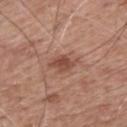Assessment: Recorded during total-body skin imaging; not selected for excision or biopsy. Background: A lesion tile, about 15 mm wide, cut from a 3D total-body photograph. A male subject, approximately 60 years of age. Captured under white-light illumination. The total-body-photography lesion software estimated an average lesion color of about L≈49 a*≈23 b*≈27 (CIELAB), a lesion–skin lightness drop of about 10, and a lesion-to-skin contrast of about 7 (normalized; higher = more distinct). The software also gave an automated nevus-likeness rating near 60 out of 100 and lesion-presence confidence of about 100/100. From the mid back. Approximately 4 mm at its widest.